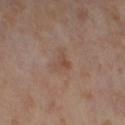No biopsy was performed on this lesion — it was imaged during a full skin examination and was not determined to be concerning.
A close-up tile cropped from a whole-body skin photograph, about 15 mm across.
This is a cross-polarized tile.
A female subject in their mid-50s.
The lesion-visualizer software estimated an average lesion color of about L≈48 a*≈18 b*≈27 (CIELAB), about 6 CIELAB-L* units darker than the surrounding skin, and a normalized border contrast of about 5.5. The software also gave border irregularity of about 5.5 on a 0–10 scale, internal color variation of about 1.5 on a 0–10 scale, and a peripheral color-asymmetry measure near 0.5.
Located on the left thigh.